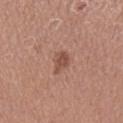The subject is a female aged around 50. Captured under white-light illumination. On the right lower leg. Longest diameter approximately 2.5 mm. A 15 mm close-up extracted from a 3D total-body photography capture.